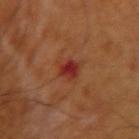Clinical impression:
No biopsy was performed on this lesion — it was imaged during a full skin examination and was not determined to be concerning.
Context:
The lesion is located on the right upper arm. A male patient, approximately 70 years of age. A lesion tile, about 15 mm wide, cut from a 3D total-body photograph. Longest diameter approximately 2.5 mm.